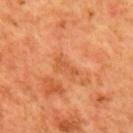Part of a total-body skin-imaging series; this lesion was reviewed on a skin check and was not flagged for biopsy. From the back. This is a cross-polarized tile. The subject is a male roughly 65 years of age. This image is a 15 mm lesion crop taken from a total-body photograph.